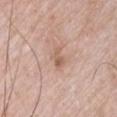The lesion was photographed on a routine skin check and not biopsied; there is no pathology result. A male subject roughly 65 years of age. Located on the right upper arm. Approximately 3 mm at its widest. Cropped from a total-body skin-imaging series; the visible field is about 15 mm.